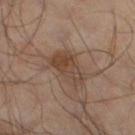Imaged during a routine full-body skin examination; the lesion was not biopsied and no histopathology is available. A close-up tile cropped from a whole-body skin photograph, about 15 mm across. The patient is a male aged around 65. The lesion's longest dimension is about 5 mm. Imaged with cross-polarized lighting. Located on the left lower leg.